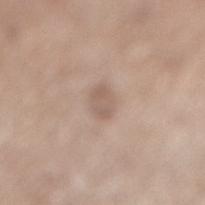Recorded during total-body skin imaging; not selected for excision or biopsy. A lesion tile, about 15 mm wide, cut from a 3D total-body photograph. A male patient, aged around 65. Captured under white-light illumination. From the right lower leg. The lesion's longest dimension is about 2.5 mm.